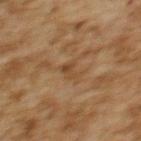biopsy status — catalogued during a skin exam; not biopsied
automated lesion analysis — a lesion area of about 3 mm²; an average lesion color of about L≈39 a*≈17 b*≈30 (CIELAB), about 6 CIELAB-L* units darker than the surrounding skin, and a normalized border contrast of about 6
image — ~15 mm crop, total-body skin-cancer survey
site — the upper back
subject — female, aged approximately 60
lesion size — ≈2.5 mm